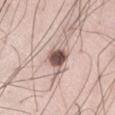* notes: imaged on a skin check; not biopsied
* patient: male, approximately 35 years of age
* anatomic site: the right thigh
* acquisition: total-body-photography crop, ~15 mm field of view
* lesion size: ~3 mm (longest diameter)
* TBP lesion metrics: a lesion–skin lightness drop of about 19 and a lesion-to-skin contrast of about 12.5 (normalized; higher = more distinct)
* lighting: white-light